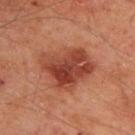| field | value |
|---|---|
| patient | male, aged approximately 65 |
| site | the back |
| image source | 15 mm crop, total-body photography |
| illumination | cross-polarized illumination |
| TBP lesion metrics | a footprint of about 21 mm², a shape eccentricity near 0.65, and a shape-asymmetry score of about 0.3 (0 = symmetric); a border-irregularity index near 3.5/10, internal color variation of about 6.5 on a 0–10 scale, and radial color variation of about 2 |
| biopsy diagnosis | an atypical melanocytic neoplasm — a lesion of indeterminate malignant potential |